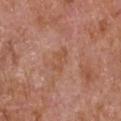The lesion is on the left upper arm.
A region of skin cropped from a whole-body photographic capture, roughly 15 mm wide.
Captured under white-light illumination.
The patient is a male aged 63 to 67.
About 3 mm across.